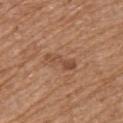Q: What lighting was used for the tile?
A: white-light illumination
Q: Who is the patient?
A: male, roughly 70 years of age
Q: Where on the body is the lesion?
A: the upper back
Q: What is the lesion's diameter?
A: ≈4 mm
Q: What kind of image is this?
A: total-body-photography crop, ~15 mm field of view